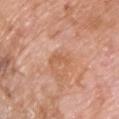Q: Was this lesion biopsied?
A: catalogued during a skin exam; not biopsied
Q: What lighting was used for the tile?
A: white-light illumination
Q: What is the anatomic site?
A: the chest
Q: What did automated image analysis measure?
A: a border-irregularity index near 6/10, internal color variation of about 0 on a 0–10 scale, and radial color variation of about 0; lesion-presence confidence of about 100/100
Q: What are the patient's age and sex?
A: male, aged around 60
Q: How large is the lesion?
A: about 3 mm
Q: How was this image acquired?
A: total-body-photography crop, ~15 mm field of view